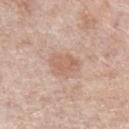Q: Is there a histopathology result?
A: catalogued during a skin exam; not biopsied
Q: What is the lesion's diameter?
A: ~3.5 mm (longest diameter)
Q: What is the imaging modality?
A: ~15 mm crop, total-body skin-cancer survey
Q: Lesion location?
A: the left forearm
Q: Patient demographics?
A: female, about 65 years old